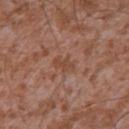notes — imaged on a skin check; not biopsied
subject — male, aged around 45
automated metrics — a lesion-detection confidence of about 100/100
illumination — white-light
size — ≈2.5 mm
anatomic site — the chest
image source — ~15 mm tile from a whole-body skin photo